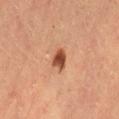The lesion was photographed on a routine skin check and not biopsied; there is no pathology result. Imaged with cross-polarized lighting. Automated image analysis of the tile measured a shape eccentricity near 0.65 and two-axis asymmetry of about 0.3. The software also gave roughly 15 lightness units darker than nearby skin and a normalized lesion–skin contrast near 11. The software also gave a border-irregularity rating of about 2.5/10 and a color-variation rating of about 2.5/10. From the lower back. The patient is a female aged 58 to 62. A roughly 15 mm field-of-view crop from a total-body skin photograph. The lesion's longest dimension is about 2.5 mm.